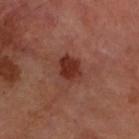Q: Was a biopsy performed?
A: imaged on a skin check; not biopsied
Q: What is the anatomic site?
A: the arm
Q: Illumination type?
A: cross-polarized
Q: Automated lesion metrics?
A: a lesion area of about 7.5 mm² and a symmetry-axis asymmetry near 0.2; a mean CIELAB color near L≈32 a*≈25 b*≈26, about 10 CIELAB-L* units darker than the surrounding skin, and a normalized border contrast of about 9; an automated nevus-likeness rating near 95 out of 100 and lesion-presence confidence of about 100/100
Q: Lesion size?
A: about 3.5 mm
Q: Who is the patient?
A: male, about 70 years old
Q: What kind of image is this?
A: ~15 mm tile from a whole-body skin photo